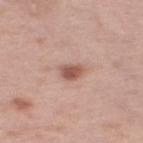No biopsy was performed on this lesion — it was imaged during a full skin examination and was not determined to be concerning.